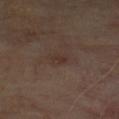The lesion is on the chest.
The subject is a male in their 70s.
This is a cross-polarized tile.
This image is a 15 mm lesion crop taken from a total-body photograph.
Approximately 3 mm at its widest.